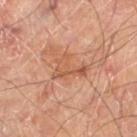<case>
<biopsy_status>not biopsied; imaged during a skin examination</biopsy_status>
<image>
  <source>total-body photography crop</source>
  <field_of_view_mm>15</field_of_view_mm>
</image>
<patient>
  <sex>male</sex>
  <age_approx>70</age_approx>
</patient>
<site>left thigh</site>
</case>